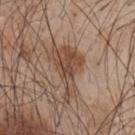Captured under white-light illumination. A male subject aged approximately 45. This image is a 15 mm lesion crop taken from a total-body photograph. Measured at roughly 6.5 mm in maximum diameter. From the mid back.